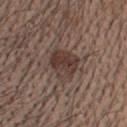  biopsy_status: not biopsied; imaged during a skin examination
  lesion_size:
    long_diameter_mm_approx: 3.5
  image:
    source: total-body photography crop
    field_of_view_mm: 15
  site: head or neck
  lighting: white-light
  patient:
    sex: male
    age_approx: 40